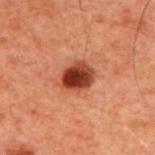This lesion was catalogued during total-body skin photography and was not selected for biopsy. A region of skin cropped from a whole-body photographic capture, roughly 15 mm wide. A male subject, aged 58–62. Captured under cross-polarized illumination. From the back. The lesion's longest dimension is about 3.5 mm.